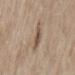The lesion was photographed on a routine skin check and not biopsied; there is no pathology result.
The subject is a female aged around 60.
Approximately 3 mm at its widest.
The total-body-photography lesion software estimated a within-lesion color-variation index near 4.5/10 and radial color variation of about 1.5. It also reported a detector confidence of about 100 out of 100 that the crop contains a lesion.
The lesion is located on the mid back.
A close-up tile cropped from a whole-body skin photograph, about 15 mm across.